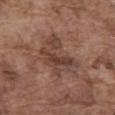Imaged during a routine full-body skin examination; the lesion was not biopsied and no histopathology is available. The lesion is on the abdomen. The tile uses white-light illumination. This image is a 15 mm lesion crop taken from a total-body photograph. Measured at roughly 6 mm in maximum diameter. A male subject, approximately 75 years of age.